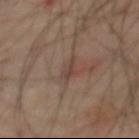  biopsy_status: not biopsied; imaged during a skin examination
  automated_metrics:
    border_irregularity_0_10: 4.5
    color_variation_0_10: 2.0
    peripheral_color_asymmetry: 0.5
  image:
    source: total-body photography crop
    field_of_view_mm: 15
  site: abdomen
  patient:
    sex: male
    age_approx: 65
  lesion_size:
    long_diameter_mm_approx: 3.5
  lighting: cross-polarized